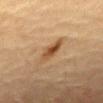Case summary:
– biopsy status — no biopsy performed (imaged during a skin exam)
– image source — ~15 mm crop, total-body skin-cancer survey
– anatomic site — the chest
– illumination — cross-polarized illumination
– lesion size — ~3.5 mm (longest diameter)
– patient — female, approximately 80 years of age